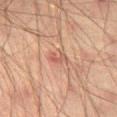biopsy status = imaged on a skin check; not biopsied
diameter = ≈2.5 mm
subject = male, roughly 45 years of age
image source = ~15 mm crop, total-body skin-cancer survey
image-analysis metrics = roughly 7 lightness units darker than nearby skin and a normalized lesion–skin contrast near 5.5; a nevus-likeness score of about 0/100 and a detector confidence of about 100 out of 100 that the crop contains a lesion
lighting = cross-polarized illumination
body site = the abdomen